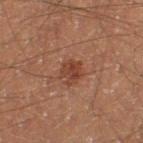The subject is a male aged 38–42. Measured at roughly 2.5 mm in maximum diameter. A region of skin cropped from a whole-body photographic capture, roughly 15 mm wide. The lesion is on the left thigh. This is a cross-polarized tile.